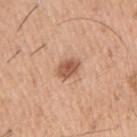Assessment: No biopsy was performed on this lesion — it was imaged during a full skin examination and was not determined to be concerning. Acquisition and patient details: The subject is a male aged around 40. Measured at roughly 2.5 mm in maximum diameter. Located on the right upper arm. This image is a 15 mm lesion crop taken from a total-body photograph.